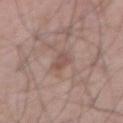Q: Was this lesion biopsied?
A: catalogued during a skin exam; not biopsied
Q: Where on the body is the lesion?
A: the front of the torso
Q: What lighting was used for the tile?
A: white-light
Q: Who is the patient?
A: male, roughly 70 years of age
Q: Automated lesion metrics?
A: an area of roughly 3.5 mm² and an eccentricity of roughly 0.75; an average lesion color of about L≈51 a*≈18 b*≈23 (CIELAB), about 8 CIELAB-L* units darker than the surrounding skin, and a lesion-to-skin contrast of about 6 (normalized; higher = more distinct); border irregularity of about 2.5 on a 0–10 scale, a color-variation rating of about 1.5/10, and radial color variation of about 0.5; a nevus-likeness score of about 0/100 and a lesion-detection confidence of about 100/100
Q: How large is the lesion?
A: about 2.5 mm
Q: How was this image acquired?
A: 15 mm crop, total-body photography